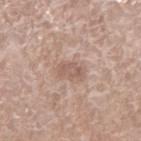lighting = white-light
acquisition = ~15 mm crop, total-body skin-cancer survey
location = the right lower leg
lesion size = about 2.5 mm
subject = female, about 75 years old